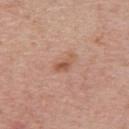| field | value |
|---|---|
| notes | no biopsy performed (imaged during a skin exam) |